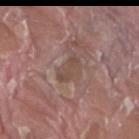Recorded during total-body skin imaging; not selected for excision or biopsy. Automated tile analysis of the lesion measured an average lesion color of about L≈47 a*≈17 b*≈22 (CIELAB). It also reported border irregularity of about 4.5 on a 0–10 scale and internal color variation of about 0.5 on a 0–10 scale. The lesion is located on the leg. Imaged with white-light lighting. A 15 mm crop from a total-body photograph taken for skin-cancer surveillance. About 3.5 mm across. A male subject, about 40 years old.